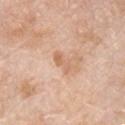Q: Was a biopsy performed?
A: total-body-photography surveillance lesion; no biopsy
Q: What is the imaging modality?
A: ~15 mm tile from a whole-body skin photo
Q: Patient demographics?
A: male, in their 60s
Q: How was the tile lit?
A: white-light
Q: Lesion location?
A: the front of the torso
Q: Lesion size?
A: about 3 mm